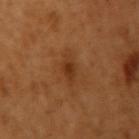No biopsy was performed on this lesion — it was imaged during a full skin examination and was not determined to be concerning. The total-body-photography lesion software estimated a mean CIELAB color near L≈36 a*≈24 b*≈36, about 7 CIELAB-L* units darker than the surrounding skin, and a normalized lesion–skin contrast near 6.5. The software also gave a color-variation rating of about 2.5/10 and a peripheral color-asymmetry measure near 0.5. A region of skin cropped from a whole-body photographic capture, roughly 15 mm wide. A female subject aged approximately 55. The lesion is on the right upper arm.